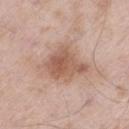notes — catalogued during a skin exam; not biopsied | tile lighting — white-light | diameter — ~4.5 mm (longest diameter) | imaging modality — 15 mm crop, total-body photography | subject — male, aged 53 to 57 | automated metrics — an eccentricity of roughly 0.6 and a shape-asymmetry score of about 0.35 (0 = symmetric); a mean CIELAB color near L≈57 a*≈20 b*≈28, a lesion–skin lightness drop of about 11, and a normalized border contrast of about 7.5; a border-irregularity index near 4/10, a color-variation rating of about 4/10, and peripheral color asymmetry of about 1.5 | site — the left thigh.